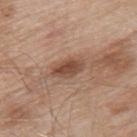  biopsy_status: not biopsied; imaged during a skin examination
  image:
    source: total-body photography crop
    field_of_view_mm: 15
  site: upper back
  patient:
    sex: male
    age_approx: 55
  lighting: white-light
  lesion_size:
    long_diameter_mm_approx: 4.0
  automated_metrics:
    cielab_L: 48
    cielab_a: 20
    cielab_b: 28
    vs_skin_darker_L: 12.0
    vs_skin_contrast_norm: 8.5
    border_irregularity_0_10: 2.5